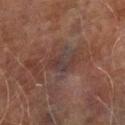Part of a total-body skin-imaging series; this lesion was reviewed on a skin check and was not flagged for biopsy. A male patient, in their mid-70s. This is a cross-polarized tile. A close-up tile cropped from a whole-body skin photograph, about 15 mm across. From the leg.